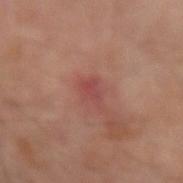Image and clinical context: This is a cross-polarized tile. A 15 mm crop from a total-body photograph taken for skin-cancer surveillance. A male patient aged approximately 65. On the arm. The recorded lesion diameter is about 2.5 mm.